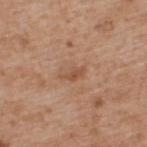{"biopsy_status": "not biopsied; imaged during a skin examination", "site": "upper back", "automated_metrics": {"eccentricity": 0.9, "nevus_likeness_0_100": 0, "lesion_detection_confidence_0_100": 100}, "lesion_size": {"long_diameter_mm_approx": 3.0}, "image": {"source": "total-body photography crop", "field_of_view_mm": 15}, "patient": {"sex": "male", "age_approx": 65}}